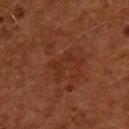Findings:
* workup · no biopsy performed (imaged during a skin exam)